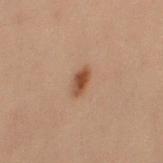Q: Was a biopsy performed?
A: total-body-photography surveillance lesion; no biopsy
Q: What is the anatomic site?
A: the arm
Q: Patient demographics?
A: female, aged around 20
Q: What kind of image is this?
A: 15 mm crop, total-body photography